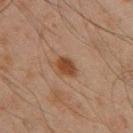A 15 mm close-up tile from a total-body photography series done for melanoma screening. The subject is a male aged 43 to 47. Measured at roughly 2.5 mm in maximum diameter. Imaged with cross-polarized lighting. The lesion is on the left thigh.